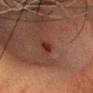Impression:
Captured during whole-body skin photography for melanoma surveillance; the lesion was not biopsied.
Clinical summary:
A lesion tile, about 15 mm wide, cut from a 3D total-body photograph. An algorithmic analysis of the crop reported a footprint of about 3 mm² and an eccentricity of roughly 0.85. The software also gave a lesion color around L≈26 a*≈22 b*≈22 in CIELAB, a lesion–skin lightness drop of about 7, and a normalized border contrast of about 8. It also reported a border-irregularity index near 2/10. Located on the head or neck. A male patient approximately 65 years of age. This is a cross-polarized tile.